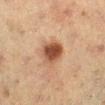The lesion was tiled from a total-body skin photograph and was not biopsied.
A 15 mm close-up tile from a total-body photography series done for melanoma screening.
Longest diameter approximately 4 mm.
An algorithmic analysis of the crop reported peripheral color asymmetry of about 1.5.
A female subject, aged approximately 55.
Captured under cross-polarized illumination.
The lesion is located on the leg.